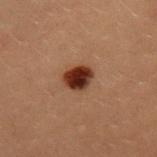workup=total-body-photography surveillance lesion; no biopsy | imaging modality=~15 mm tile from a whole-body skin photo | site=the upper back | subject=female, aged 18–22 | diameter=about 3.5 mm.